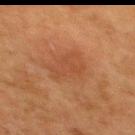Q: Was a biopsy performed?
A: total-body-photography surveillance lesion; no biopsy
Q: What is the imaging modality?
A: total-body-photography crop, ~15 mm field of view
Q: What lighting was used for the tile?
A: cross-polarized illumination
Q: Patient demographics?
A: female, in their 50s
Q: What is the lesion's diameter?
A: ≈5 mm
Q: Where on the body is the lesion?
A: the upper back
Q: What did automated image analysis measure?
A: an area of roughly 11 mm², an outline eccentricity of about 0.85 (0 = round, 1 = elongated), and a symmetry-axis asymmetry near 0.25; a border-irregularity index near 3.5/10, a color-variation rating of about 2/10, and peripheral color asymmetry of about 0.5; a lesion-detection confidence of about 100/100